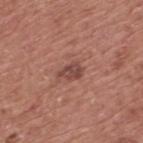Clinical impression:
No biopsy was performed on this lesion — it was imaged during a full skin examination and was not determined to be concerning.
Clinical summary:
The patient is a male aged approximately 75. Imaged with white-light lighting. A 15 mm close-up extracted from a 3D total-body photography capture. Located on the back.